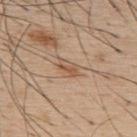follow-up: imaged on a skin check; not biopsied | body site: the upper back | automated metrics: an outline eccentricity of about 0.85 (0 = round, 1 = elongated) and a symmetry-axis asymmetry near 0.25; a border-irregularity rating of about 2.5/10 | patient: male, aged around 55 | lesion diameter: ~2.5 mm (longest diameter) | imaging modality: 15 mm crop, total-body photography.